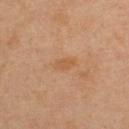<tbp_lesion>
<biopsy_status>not biopsied; imaged during a skin examination</biopsy_status>
<site>upper back</site>
<lighting>cross-polarized</lighting>
<image>
  <source>total-body photography crop</source>
  <field_of_view_mm>15</field_of_view_mm>
</image>
<patient>
  <sex>female</sex>
  <age_approx>45</age_approx>
</patient>
<automated_metrics>
  <eccentricity>0.85</eccentricity>
  <shape_asymmetry>0.15</shape_asymmetry>
  <vs_skin_darker_L>5.0</vs_skin_darker_L>
  <vs_skin_contrast_norm>5.0</vs_skin_contrast_norm>
  <border_irregularity_0_10>1.5</border_irregularity_0_10>
  <color_variation_0_10>2.0</color_variation_0_10>
  <peripheral_color_asymmetry>1.0</peripheral_color_asymmetry>
</automated_metrics>
<lesion_size>
  <long_diameter_mm_approx>3.0</long_diameter_mm_approx>
</lesion_size>
</tbp_lesion>